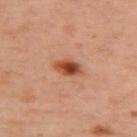Longest diameter approximately 3.5 mm. Located on the upper back. A 15 mm crop from a total-body photograph taken for skin-cancer surveillance. A female subject, in their 40s.